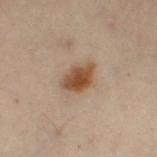Part of a total-body skin-imaging series; this lesion was reviewed on a skin check and was not flagged for biopsy.
The lesion is on the right thigh.
The patient is a female aged 48 to 52.
A region of skin cropped from a whole-body photographic capture, roughly 15 mm wide.